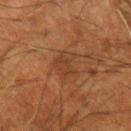Clinical impression: Captured during whole-body skin photography for melanoma surveillance; the lesion was not biopsied. Clinical summary: The recorded lesion diameter is about 3.5 mm. Cropped from a total-body skin-imaging series; the visible field is about 15 mm. On the left forearm. A male patient, aged 63–67.